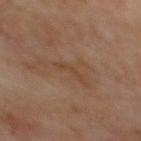The lesion was photographed on a routine skin check and not biopsied; there is no pathology result.
A male subject aged around 70.
From the mid back.
The tile uses cross-polarized illumination.
Measured at roughly 3 mm in maximum diameter.
A region of skin cropped from a whole-body photographic capture, roughly 15 mm wide.